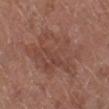Case summary:
– follow-up · catalogued during a skin exam; not biopsied
– body site · the right lower leg
– patient · female, aged 78–82
– image source · ~15 mm tile from a whole-body skin photo
– automated metrics · a mean CIELAB color near L≈45 a*≈21 b*≈26, about 6 CIELAB-L* units darker than the surrounding skin, and a normalized lesion–skin contrast near 5; border irregularity of about 5.5 on a 0–10 scale, internal color variation of about 3.5 on a 0–10 scale, and a peripheral color-asymmetry measure near 1; a classifier nevus-likeness of about 0/100 and lesion-presence confidence of about 100/100
– tile lighting · white-light illumination